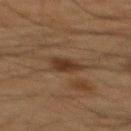Recorded during total-body skin imaging; not selected for excision or biopsy. A male patient, aged around 65. This is a cross-polarized tile. A region of skin cropped from a whole-body photographic capture, roughly 15 mm wide. About 3.5 mm across. The lesion is located on the mid back.